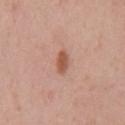site: the front of the torso; subject: male, approximately 55 years of age; lesion diameter: ~3 mm (longest diameter); image source: total-body-photography crop, ~15 mm field of view.